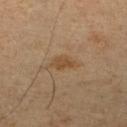No biopsy was performed on this lesion — it was imaged during a full skin examination and was not determined to be concerning.
A close-up tile cropped from a whole-body skin photograph, about 15 mm across.
The patient is a male in their mid-50s.
The lesion is located on the right lower leg.